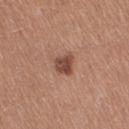Findings:
– subject: female, aged around 50
– site: the leg
– imaging modality: ~15 mm crop, total-body skin-cancer survey
– lesion diameter: ~2.5 mm (longest diameter)